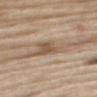Case summary:
* workup · catalogued during a skin exam; not biopsied
* patient · male, aged 68–72
* illumination · white-light illumination
* lesion diameter · ≈7 mm
* location · the right thigh
* image source · ~15 mm crop, total-body skin-cancer survey